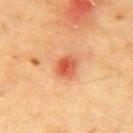Clinical impression: No biopsy was performed on this lesion — it was imaged during a full skin examination and was not determined to be concerning. Context: Automated tile analysis of the lesion measured a mean CIELAB color near L≈51 a*≈31 b*≈35 and a normalized border contrast of about 8. Located on the chest. Captured under cross-polarized illumination. This image is a 15 mm lesion crop taken from a total-body photograph. The patient is a male in their mid-80s or older.